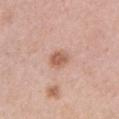{"biopsy_status": "not biopsied; imaged during a skin examination", "site": "chest", "image": {"source": "total-body photography crop", "field_of_view_mm": 15}, "patient": {"sex": "female", "age_approx": 60}}